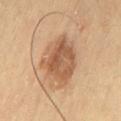Q: Is there a histopathology result?
A: catalogued during a skin exam; not biopsied
Q: What lighting was used for the tile?
A: cross-polarized
Q: What are the patient's age and sex?
A: male, in their mid- to late 60s
Q: What is the lesion's diameter?
A: ≈5.5 mm
Q: What kind of image is this?
A: ~15 mm tile from a whole-body skin photo
Q: Lesion location?
A: the leg